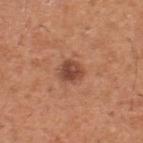Part of a total-body skin-imaging series; this lesion was reviewed on a skin check and was not flagged for biopsy.